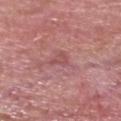workup: catalogued during a skin exam; not biopsied | acquisition: ~15 mm tile from a whole-body skin photo | subject: male, in their mid-60s | illumination: white-light illumination | size: ~2.5 mm (longest diameter) | site: the head or neck.